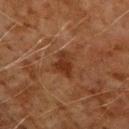Q: Was a biopsy performed?
A: no biopsy performed (imaged during a skin exam)
Q: What is the imaging modality?
A: 15 mm crop, total-body photography
Q: Lesion size?
A: about 3.5 mm
Q: Where on the body is the lesion?
A: the right upper arm
Q: What are the patient's age and sex?
A: male, approximately 70 years of age